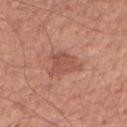follow-up: total-body-photography surveillance lesion; no biopsy
automated metrics: an area of roughly 8 mm², a shape eccentricity near 0.6, and a symmetry-axis asymmetry near 0.45; a border-irregularity rating of about 5/10 and peripheral color asymmetry of about 1; an automated nevus-likeness rating near 15 out of 100 and a detector confidence of about 100 out of 100 that the crop contains a lesion
tile lighting: white-light illumination
patient: male, aged 53 to 57
anatomic site: the left upper arm
imaging modality: 15 mm crop, total-body photography